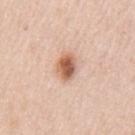Case summary:
* body site — the left upper arm
* size — ~3.5 mm (longest diameter)
* subject — female, about 45 years old
* imaging modality — 15 mm crop, total-body photography
* illumination — white-light illumination